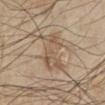biopsy_status: not biopsied; imaged during a skin examination
site: left lower leg
image:
  source: total-body photography crop
  field_of_view_mm: 15
lesion_size:
  long_diameter_mm_approx: 4.5
patient:
  sex: male
  age_approx: 55
automated_metrics:
  area_mm2_approx: 12.0
  shape_asymmetry: 0.3
  cielab_L: 54
  cielab_a: 14
  cielab_b: 28
  vs_skin_darker_L: 8.0
  vs_skin_contrast_norm: 5.5
  border_irregularity_0_10: 4.5
  peripheral_color_asymmetry: 1.5